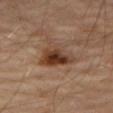biopsy_status: not biopsied; imaged during a skin examination
site: right thigh
lesion_size:
  long_diameter_mm_approx: 4.5
lighting: cross-polarized
image:
  source: total-body photography crop
  field_of_view_mm: 15
patient:
  sex: male
  age_approx: 70
automated_metrics:
  eccentricity: 0.7
  shape_asymmetry: 0.35
  border_irregularity_0_10: 3.5
  color_variation_0_10: 8.0
  lesion_detection_confidence_0_100: 100